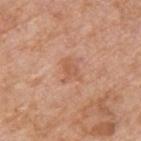Recorded during total-body skin imaging; not selected for excision or biopsy. A 15 mm crop from a total-body photograph taken for skin-cancer surveillance. A male patient roughly 55 years of age. This is a white-light tile. The lesion is located on the upper back.